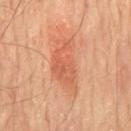Recorded during total-body skin imaging; not selected for excision or biopsy. Approximately 7 mm at its widest. The patient is a male aged 63 to 67. A close-up tile cropped from a whole-body skin photograph, about 15 mm across. Imaged with cross-polarized lighting. The lesion is located on the chest.